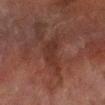The lesion was photographed on a routine skin check and not biopsied; there is no pathology result.
A male patient roughly 70 years of age.
This is a cross-polarized tile.
Cropped from a total-body skin-imaging series; the visible field is about 15 mm.
The recorded lesion diameter is about 5 mm.
The lesion is on the leg.